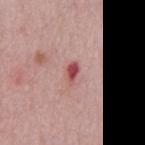subject: male, roughly 50 years of age; site: the chest; lesion diameter: ≈2.5 mm; illumination: white-light; image: total-body-photography crop, ~15 mm field of view.